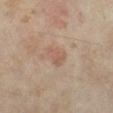A female subject, aged 33–37. The recorded lesion diameter is about 3 mm. The lesion is on the right lower leg. Captured under cross-polarized illumination. A region of skin cropped from a whole-body photographic capture, roughly 15 mm wide. Automated image analysis of the tile measured a lesion area of about 4.5 mm², an eccentricity of roughly 0.7, and two-axis asymmetry of about 0.35. The analysis additionally found a lesion–skin lightness drop of about 6 and a normalized lesion–skin contrast near 5. It also reported a border-irregularity index near 3/10 and peripheral color asymmetry of about 0.5. The analysis additionally found an automated nevus-likeness rating near 20 out of 100 and a lesion-detection confidence of about 100/100.